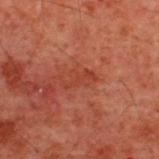Assessment:
Recorded during total-body skin imaging; not selected for excision or biopsy.
Image and clinical context:
This is a cross-polarized tile. Located on the upper back. Measured at roughly 3.5 mm in maximum diameter. A male patient aged approximately 60. A close-up tile cropped from a whole-body skin photograph, about 15 mm across.